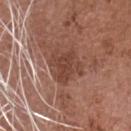The lesion was photographed on a routine skin check and not biopsied; there is no pathology result.
A region of skin cropped from a whole-body photographic capture, roughly 15 mm wide.
The lesion is on the head or neck.
The subject is a male aged 73 to 77.